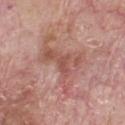Q: Was this lesion biopsied?
A: no biopsy performed (imaged during a skin exam)
Q: What kind of image is this?
A: total-body-photography crop, ~15 mm field of view
Q: What did automated image analysis measure?
A: a shape eccentricity near 0.75 and two-axis asymmetry of about 0.55; about 8 CIELAB-L* units darker than the surrounding skin and a normalized lesion–skin contrast near 5.5; a classifier nevus-likeness of about 0/100
Q: What is the anatomic site?
A: the chest
Q: Lesion size?
A: ≈7.5 mm
Q: Patient demographics?
A: male, aged 63–67
Q: What lighting was used for the tile?
A: white-light